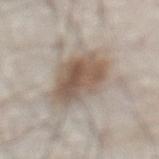Clinical impression: No biopsy was performed on this lesion — it was imaged during a full skin examination and was not determined to be concerning. Acquisition and patient details: A male subject, aged around 80. The lesion is on the abdomen. Measured at roughly 5.5 mm in maximum diameter. The tile uses white-light illumination. A close-up tile cropped from a whole-body skin photograph, about 15 mm across.